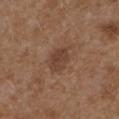Part of a total-body skin-imaging series; this lesion was reviewed on a skin check and was not flagged for biopsy. On the right upper arm. A female patient aged around 35. A 15 mm close-up tile from a total-body photography series done for melanoma screening. Longest diameter approximately 3.5 mm. The total-body-photography lesion software estimated an area of roughly 6 mm², an eccentricity of roughly 0.65, and a shape-asymmetry score of about 0.15 (0 = symmetric). Captured under white-light illumination.